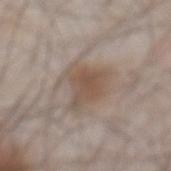Impression:
Recorded during total-body skin imaging; not selected for excision or biopsy.
Clinical summary:
A 15 mm close-up extracted from a 3D total-body photography capture. A male patient aged 53–57. Located on the front of the torso. The recorded lesion diameter is about 5 mm. The tile uses white-light illumination.